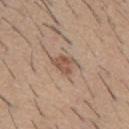Assessment: Imaged during a routine full-body skin examination; the lesion was not biopsied and no histopathology is available. Acquisition and patient details: The patient is a male aged around 60. The lesion is located on the mid back. A lesion tile, about 15 mm wide, cut from a 3D total-body photograph.